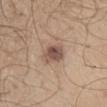Recorded during total-body skin imaging; not selected for excision or biopsy.
The subject is a male about 55 years old.
Located on the chest.
Automated image analysis of the tile measured a border-irregularity index near 2/10, internal color variation of about 5 on a 0–10 scale, and peripheral color asymmetry of about 2.
A lesion tile, about 15 mm wide, cut from a 3D total-body photograph.
The lesion's longest dimension is about 3 mm.
The tile uses white-light illumination.